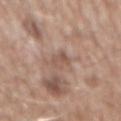The lesion-visualizer software estimated an average lesion color of about L≈53 a*≈19 b*≈26 (CIELAB), about 8 CIELAB-L* units darker than the surrounding skin, and a lesion-to-skin contrast of about 6 (normalized; higher = more distinct). And it measured a border-irregularity rating of about 3.5/10. A roughly 15 mm field-of-view crop from a total-body skin photograph. This is a white-light tile. On the abdomen. The patient is a male in their 60s. The recorded lesion diameter is about 3 mm.